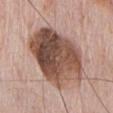{"biopsy_status": "not biopsied; imaged during a skin examination", "automated_metrics": {"area_mm2_approx": 42.0, "eccentricity": 0.7, "border_irregularity_0_10": 2.0, "color_variation_0_10": 9.5, "peripheral_color_asymmetry": 4.0, "nevus_likeness_0_100": 5, "lesion_detection_confidence_0_100": 100}, "lesion_size": {"long_diameter_mm_approx": 9.0}, "site": "chest", "image": {"source": "total-body photography crop", "field_of_view_mm": 15}, "patient": {"sex": "male", "age_approx": 70}}